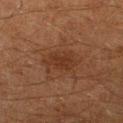Notes:
– workup: no biopsy performed (imaged during a skin exam)
– body site: the right lower leg
– image: 15 mm crop, total-body photography
– tile lighting: cross-polarized
– subject: male, aged 58–62